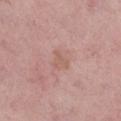This lesion was catalogued during total-body skin photography and was not selected for biopsy. Cropped from a total-body skin-imaging series; the visible field is about 15 mm. A female subject in their 50s. An algorithmic analysis of the crop reported an area of roughly 3 mm², an eccentricity of roughly 0.6, and a shape-asymmetry score of about 0.5 (0 = symmetric). The software also gave a normalized border contrast of about 4.5. It also reported border irregularity of about 5 on a 0–10 scale, a color-variation rating of about 0.5/10, and peripheral color asymmetry of about 0. It also reported a classifier nevus-likeness of about 0/100. Located on the right lower leg.